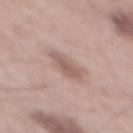{"biopsy_status": "not biopsied; imaged during a skin examination", "site": "mid back", "lighting": "white-light", "image": {"source": "total-body photography crop", "field_of_view_mm": 15}, "automated_metrics": {"area_mm2_approx": 5.5, "eccentricity": 0.9, "shape_asymmetry": 0.25, "nevus_likeness_0_100": 10, "lesion_detection_confidence_0_100": 100}, "lesion_size": {"long_diameter_mm_approx": 4.0}, "patient": {"sex": "male", "age_approx": 55}}